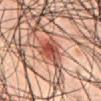Assessment: This lesion was catalogued during total-body skin photography and was not selected for biopsy. Image and clinical context: The patient is a male aged 43–47. The recorded lesion diameter is about 4 mm. Cropped from a whole-body photographic skin survey; the tile spans about 15 mm. This is a cross-polarized tile. The lesion is located on the abdomen.